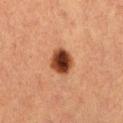Part of a total-body skin-imaging series; this lesion was reviewed on a skin check and was not flagged for biopsy. The patient is a female aged 38–42. From the left thigh. A close-up tile cropped from a whole-body skin photograph, about 15 mm across.